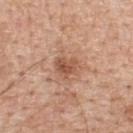| feature | finding |
|---|---|
| location | the upper back |
| patient | male, in their 60s |
| automated lesion analysis | a lesion color around L≈54 a*≈23 b*≈32 in CIELAB, a lesion–skin lightness drop of about 10, and a normalized border contrast of about 7; a border-irregularity index near 3/10 and internal color variation of about 4 on a 0–10 scale; a classifier nevus-likeness of about 10/100 and lesion-presence confidence of about 100/100 |
| size | ~3 mm (longest diameter) |
| lighting | white-light illumination |
| image source | 15 mm crop, total-body photography |